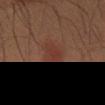Assessment:
No biopsy was performed on this lesion — it was imaged during a full skin examination and was not determined to be concerning.
Clinical summary:
This image is a 15 mm lesion crop taken from a total-body photograph. The subject is a male aged 58–62. The tile uses cross-polarized illumination. From the right thigh. The lesion's longest dimension is about 3.5 mm.